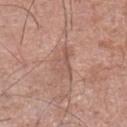Clinical impression: This lesion was catalogued during total-body skin photography and was not selected for biopsy. Clinical summary: The lesion's longest dimension is about 4 mm. Automated image analysis of the tile measured border irregularity of about 5.5 on a 0–10 scale and a within-lesion color-variation index near 2/10. Imaged with white-light lighting. Cropped from a total-body skin-imaging series; the visible field is about 15 mm. The patient is a male roughly 60 years of age. From the left lower leg.